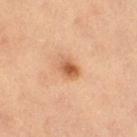The lesion was tiled from a total-body skin photograph and was not biopsied.
Cropped from a total-body skin-imaging series; the visible field is about 15 mm.
Located on the right thigh.
Longest diameter approximately 3 mm.
A female patient, in their mid- to late 30s.